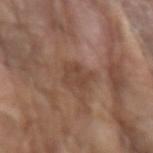Captured during whole-body skin photography for melanoma surveillance; the lesion was not biopsied.
A male subject in their 80s.
From the left forearm.
A roughly 15 mm field-of-view crop from a total-body skin photograph.
Captured under white-light illumination.